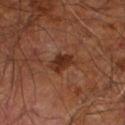Q: Lesion size?
A: about 3 mm
Q: What is the anatomic site?
A: the right leg
Q: What lighting was used for the tile?
A: cross-polarized illumination
Q: Who is the patient?
A: male, roughly 60 years of age
Q: How was this image acquired?
A: total-body-photography crop, ~15 mm field of view